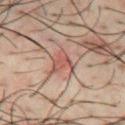Case summary:
– notes: total-body-photography surveillance lesion; no biopsy
– lesion size: about 3 mm
– image: total-body-photography crop, ~15 mm field of view
– body site: the chest
– tile lighting: cross-polarized illumination
– subject: male, about 40 years old